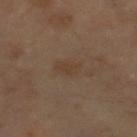A close-up tile cropped from a whole-body skin photograph, about 15 mm across. A male subject, aged approximately 60. On the right lower leg.